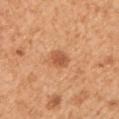The lesion was tiled from a total-body skin photograph and was not biopsied. A male patient about 55 years old. An algorithmic analysis of the crop reported an average lesion color of about L≈56 a*≈27 b*≈38 (CIELAB) and a normalized lesion–skin contrast near 7. It also reported border irregularity of about 1.5 on a 0–10 scale, a color-variation rating of about 2/10, and peripheral color asymmetry of about 0.5. It also reported a nevus-likeness score of about 80/100. A region of skin cropped from a whole-body photographic capture, roughly 15 mm wide. From the arm. Imaged with white-light lighting.